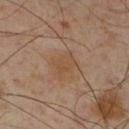Notes:
• workup: catalogued during a skin exam; not biopsied
• site: the right lower leg
• image source: 15 mm crop, total-body photography
• lesion size: ~3.5 mm (longest diameter)
• patient: male, approximately 55 years of age